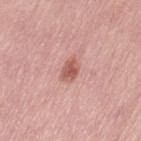The lesion was tiled from a total-body skin photograph and was not biopsied.